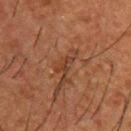Clinical impression: This lesion was catalogued during total-body skin photography and was not selected for biopsy.